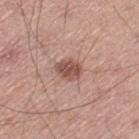Impression: Part of a total-body skin-imaging series; this lesion was reviewed on a skin check and was not flagged for biopsy. Clinical summary: Cropped from a whole-body photographic skin survey; the tile spans about 15 mm. This is a white-light tile. The lesion is located on the left thigh. Approximately 3 mm at its widest. The subject is a male approximately 50 years of age.